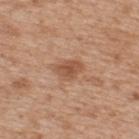workup: catalogued during a skin exam; not biopsied
patient: male, in their mid-60s
acquisition: ~15 mm tile from a whole-body skin photo
site: the upper back
tile lighting: white-light illumination
lesion size: ≈3 mm